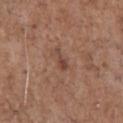Imaged during a routine full-body skin examination; the lesion was not biopsied and no histopathology is available. The tile uses white-light illumination. A male patient, about 70 years old. This image is a 15 mm lesion crop taken from a total-body photograph. Located on the front of the torso.